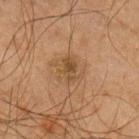{
  "biopsy_status": "not biopsied; imaged during a skin examination",
  "automated_metrics": {
    "cielab_L": 38,
    "cielab_a": 14,
    "cielab_b": 29,
    "nevus_likeness_0_100": 15
  },
  "site": "arm",
  "patient": {
    "sex": "male",
    "age_approx": 65
  },
  "lesion_size": {
    "long_diameter_mm_approx": 3.0
  },
  "image": {
    "source": "total-body photography crop",
    "field_of_view_mm": 15
  }
}